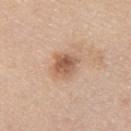Q: Was this lesion biopsied?
A: no biopsy performed (imaged during a skin exam)
Q: What lighting was used for the tile?
A: white-light illumination
Q: What is the lesion's diameter?
A: ≈3 mm
Q: What is the imaging modality?
A: ~15 mm crop, total-body skin-cancer survey
Q: Lesion location?
A: the upper back
Q: What are the patient's age and sex?
A: female, in their mid- to late 50s
Q: Automated lesion metrics?
A: an eccentricity of roughly 0.4 and a shape-asymmetry score of about 0.2 (0 = symmetric); an average lesion color of about L≈58 a*≈20 b*≈32 (CIELAB) and a lesion-to-skin contrast of about 8 (normalized; higher = more distinct); border irregularity of about 2 on a 0–10 scale, a within-lesion color-variation index near 5/10, and peripheral color asymmetry of about 1.5; a classifier nevus-likeness of about 40/100